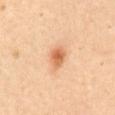Recorded during total-body skin imaging; not selected for excision or biopsy. The tile uses cross-polarized illumination. Automated image analysis of the tile measured a mean CIELAB color near L≈68 a*≈26 b*≈40, roughly 13 lightness units darker than nearby skin, and a lesion-to-skin contrast of about 8 (normalized; higher = more distinct). And it measured border irregularity of about 1.5 on a 0–10 scale and peripheral color asymmetry of about 1.5. The lesion is located on the abdomen. A 15 mm crop from a total-body photograph taken for skin-cancer surveillance. A female patient about 55 years old. Measured at roughly 3 mm in maximum diameter.